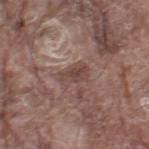Impression:
Captured during whole-body skin photography for melanoma surveillance; the lesion was not biopsied.
Background:
Cropped from a total-body skin-imaging series; the visible field is about 15 mm. An algorithmic analysis of the crop reported a footprint of about 6 mm² and a symmetry-axis asymmetry near 0.25. And it measured a border-irregularity rating of about 2.5/10, internal color variation of about 3.5 on a 0–10 scale, and radial color variation of about 1. From the right thigh. Longest diameter approximately 3.5 mm. The tile uses white-light illumination. A male patient aged around 80.